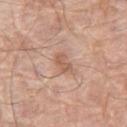Findings:
* biopsy status: total-body-photography surveillance lesion; no biopsy
* body site: the right thigh
* lesion size: about 2.5 mm
* acquisition: ~15 mm tile from a whole-body skin photo
* automated metrics: a footprint of about 4 mm², a shape eccentricity near 0.75, and a symmetry-axis asymmetry near 0.25; a nevus-likeness score of about 10/100 and a lesion-detection confidence of about 100/100
* subject: male, about 80 years old